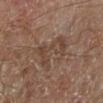Clinical impression: This lesion was catalogued during total-body skin photography and was not selected for biopsy. Image and clinical context: The lesion is on the left lower leg. This is a cross-polarized tile. A region of skin cropped from a whole-body photographic capture, roughly 15 mm wide. A male patient, roughly 70 years of age.